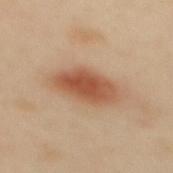Acquisition and patient details: A roughly 15 mm field-of-view crop from a total-body skin photograph. The subject is a female about 40 years old. On the mid back. An algorithmic analysis of the crop reported a border-irregularity rating of about 2/10, a color-variation rating of about 5/10, and radial color variation of about 1.5. The software also gave a classifier nevus-likeness of about 100/100 and a lesion-detection confidence of about 100/100. Measured at roughly 6 mm in maximum diameter. This is a cross-polarized tile.